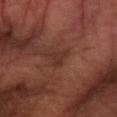The lesion was photographed on a routine skin check and not biopsied; there is no pathology result.
An algorithmic analysis of the crop reported an average lesion color of about L≈30 a*≈21 b*≈24 (CIELAB), a lesion–skin lightness drop of about 6, and a normalized border contrast of about 5.5. The analysis additionally found a lesion-detection confidence of about 60/100.
About 2.5 mm across.
The lesion is located on the right forearm.
Captured under cross-polarized illumination.
This image is a 15 mm lesion crop taken from a total-body photograph.
A male subject aged 68–72.